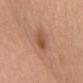Recorded during total-body skin imaging; not selected for excision or biopsy. A female patient aged 58 to 62. Imaged with white-light lighting. The lesion is located on the arm. A region of skin cropped from a whole-body photographic capture, roughly 15 mm wide. Longest diameter approximately 3 mm.